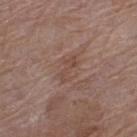No biopsy was performed on this lesion — it was imaged during a full skin examination and was not determined to be concerning. The lesion is on the left thigh. The total-body-photography lesion software estimated a footprint of about 4 mm², a shape eccentricity near 0.9, and a shape-asymmetry score of about 0.35 (0 = symmetric). The software also gave a border-irregularity rating of about 5/10, a color-variation rating of about 0.5/10, and peripheral color asymmetry of about 0. The analysis additionally found an automated nevus-likeness rating near 0 out of 100 and a detector confidence of about 100 out of 100 that the crop contains a lesion. The patient is a female aged 68 to 72. Approximately 3.5 mm at its widest. Imaged with white-light lighting. A roughly 15 mm field-of-view crop from a total-body skin photograph.